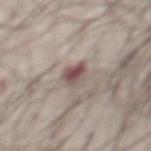Assessment:
The lesion was tiled from a total-body skin photograph and was not biopsied.
Context:
The recorded lesion diameter is about 3 mm. The patient is a male about 70 years old. The total-body-photography lesion software estimated a lesion area of about 5.5 mm², a shape eccentricity near 0.6, and two-axis asymmetry of about 0.2. It also reported a border-irregularity rating of about 2/10 and a within-lesion color-variation index near 7.5/10. The analysis additionally found an automated nevus-likeness rating near 10 out of 100 and a lesion-detection confidence of about 95/100. A 15 mm close-up tile from a total-body photography series done for melanoma screening. From the abdomen.